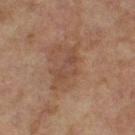workup — imaged on a skin check; not biopsied
patient — female, aged 58 to 62
tile lighting — cross-polarized
image source — 15 mm crop, total-body photography
location — the right thigh